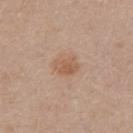| field | value |
|---|---|
| workup | total-body-photography surveillance lesion; no biopsy |
| tile lighting | white-light |
| subject | female, aged 38–42 |
| image source | 15 mm crop, total-body photography |
| diameter | ~2.5 mm (longest diameter) |
| location | the back |